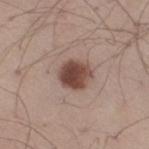Findings:
• workup · no biopsy performed (imaged during a skin exam)
• image · ~15 mm tile from a whole-body skin photo
• anatomic site · the right thigh
• patient · male, aged 28 to 32